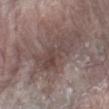{
  "biopsy_status": "not biopsied; imaged during a skin examination",
  "image": {
    "source": "total-body photography crop",
    "field_of_view_mm": 15
  },
  "site": "left forearm",
  "lesion_size": {
    "long_diameter_mm_approx": 6.0
  },
  "patient": {
    "sex": "male",
    "age_approx": 80
  },
  "automated_metrics": {
    "area_mm2_approx": 16.0,
    "eccentricity": 0.85,
    "cielab_L": 44,
    "cielab_a": 15,
    "cielab_b": 18,
    "vs_skin_darker_L": 8.0,
    "vs_skin_contrast_norm": 6.5,
    "border_irregularity_0_10": 4.5,
    "peripheral_color_asymmetry": 1.5,
    "nevus_likeness_0_100": 0,
    "lesion_detection_confidence_0_100": 60
  }
}